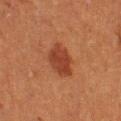automated metrics = an average lesion color of about L≈33 a*≈24 b*≈29 (CIELAB), a lesion–skin lightness drop of about 9, and a normalized lesion–skin contrast near 8; a color-variation rating of about 2.5/10 and radial color variation of about 1 | tile lighting = cross-polarized illumination | image = ~15 mm crop, total-body skin-cancer survey | subject = female, aged around 40 | size = about 4 mm | location = the right thigh.